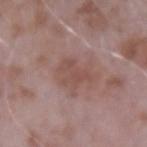Imaged during a routine full-body skin examination; the lesion was not biopsied and no histopathology is available. Approximately 4 mm at its widest. A male subject, aged 63 to 67. This is a white-light tile. A 15 mm close-up tile from a total-body photography series done for melanoma screening. The lesion is on the arm.